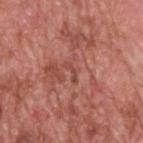Imaged during a routine full-body skin examination; the lesion was not biopsied and no histopathology is available. The lesion is on the upper back. A close-up tile cropped from a whole-body skin photograph, about 15 mm across. A male patient, aged approximately 60. Imaged with white-light lighting. Longest diameter approximately 2.5 mm.